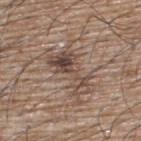Q: Is there a histopathology result?
A: imaged on a skin check; not biopsied
Q: What are the patient's age and sex?
A: male, about 65 years old
Q: What is the imaging modality?
A: total-body-photography crop, ~15 mm field of view
Q: Where on the body is the lesion?
A: the upper back